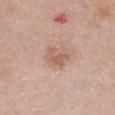| key | value |
|---|---|
| biopsy status | imaged on a skin check; not biopsied |
| image-analysis metrics | a nevus-likeness score of about 15/100 and a detector confidence of about 100 out of 100 that the crop contains a lesion |
| illumination | white-light |
| acquisition | 15 mm crop, total-body photography |
| size | about 3 mm |
| patient | female, aged around 50 |
| location | the chest |